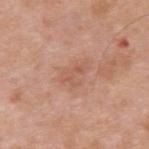Recorded during total-body skin imaging; not selected for excision or biopsy.
A 15 mm crop from a total-body photograph taken for skin-cancer surveillance.
The lesion is located on the upper back.
An algorithmic analysis of the crop reported a footprint of about 4.5 mm², a shape eccentricity near 0.8, and a shape-asymmetry score of about 0.45 (0 = symmetric).
Measured at roughly 3.5 mm in maximum diameter.
Captured under white-light illumination.
A male patient in their mid-30s.